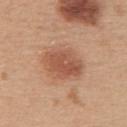Assessment:
Recorded during total-body skin imaging; not selected for excision or biopsy.
Context:
A female patient, in their mid-40s. The total-body-photography lesion software estimated an area of roughly 14 mm², an outline eccentricity of about 0.7 (0 = round, 1 = elongated), and a shape-asymmetry score of about 0.15 (0 = symmetric). The software also gave an automated nevus-likeness rating near 95 out of 100 and lesion-presence confidence of about 100/100. The lesion is located on the back. The tile uses white-light illumination. Cropped from a whole-body photographic skin survey; the tile spans about 15 mm.